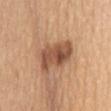Automated image analysis of the tile measured a classifier nevus-likeness of about 50/100. From the chest. A lesion tile, about 15 mm wide, cut from a 3D total-body photograph. A female subject, aged 63 to 67. Imaged with white-light lighting.